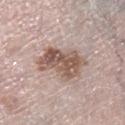Assessment:
This lesion was catalogued during total-body skin photography and was not selected for biopsy.
Acquisition and patient details:
A female patient in their mid-60s. This is a white-light tile. A roughly 15 mm field-of-view crop from a total-body skin photograph. The lesion's longest dimension is about 6.5 mm. An algorithmic analysis of the crop reported roughly 13 lightness units darker than nearby skin and a normalized border contrast of about 9. And it measured border irregularity of about 4 on a 0–10 scale and a peripheral color-asymmetry measure near 2.5. The software also gave a classifier nevus-likeness of about 90/100 and lesion-presence confidence of about 100/100. Located on the right lower leg.